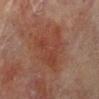A female patient aged approximately 80. This image is a 15 mm lesion crop taken from a total-body photograph. The tile uses cross-polarized illumination. The lesion is on the left lower leg.